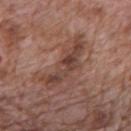A 15 mm close-up tile from a total-body photography series done for melanoma screening. A male subject aged around 70. On the mid back.